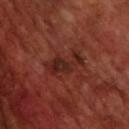Q: Was this lesion biopsied?
A: catalogued during a skin exam; not biopsied
Q: What kind of image is this?
A: total-body-photography crop, ~15 mm field of view
Q: Lesion location?
A: the upper back
Q: Who is the patient?
A: male, in their 70s
Q: What did automated image analysis measure?
A: a symmetry-axis asymmetry near 0.5; a mean CIELAB color near L≈24 a*≈24 b*≈24, about 8 CIELAB-L* units darker than the surrounding skin, and a normalized border contrast of about 8; a border-irregularity index near 8/10, internal color variation of about 3 on a 0–10 scale, and peripheral color asymmetry of about 0.5
Q: How was the tile lit?
A: cross-polarized illumination
Q: Lesion size?
A: about 5 mm